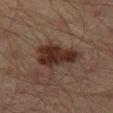A 15 mm crop from a total-body photograph taken for skin-cancer surveillance. The subject is a male in their mid-40s. Imaged with cross-polarized lighting. An algorithmic analysis of the crop reported an automated nevus-likeness rating near 65 out of 100 and lesion-presence confidence of about 100/100. The lesion is located on the left thigh.